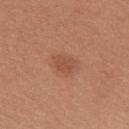Captured during whole-body skin photography for melanoma surveillance; the lesion was not biopsied.
A female patient approximately 25 years of age.
Measured at roughly 3.5 mm in maximum diameter.
The total-body-photography lesion software estimated radial color variation of about 0.5.
From the upper back.
A 15 mm close-up tile from a total-body photography series done for melanoma screening.
Captured under white-light illumination.